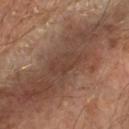Q: Was a biopsy performed?
A: imaged on a skin check; not biopsied
Q: What kind of image is this?
A: ~15 mm tile from a whole-body skin photo
Q: Automated lesion metrics?
A: a lesion area of about 6 mm² and an eccentricity of roughly 0.9; a border-irregularity index near 5/10 and a color-variation rating of about 2/10; a classifier nevus-likeness of about 0/100 and a lesion-detection confidence of about 60/100
Q: How was the tile lit?
A: cross-polarized
Q: How large is the lesion?
A: about 4.5 mm
Q: Where on the body is the lesion?
A: the left forearm